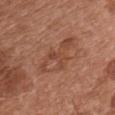Recorded during total-body skin imaging; not selected for excision or biopsy.
Located on the chest.
The recorded lesion diameter is about 7 mm.
Captured under white-light illumination.
A roughly 15 mm field-of-view crop from a total-body skin photograph.
A female subject, roughly 65 years of age.
An algorithmic analysis of the crop reported a lesion color around L≈47 a*≈23 b*≈30 in CIELAB and a lesion-to-skin contrast of about 5 (normalized; higher = more distinct). The software also gave a border-irregularity rating of about 5/10. And it measured an automated nevus-likeness rating near 0 out of 100 and lesion-presence confidence of about 100/100.